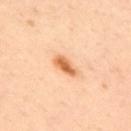follow-up = catalogued during a skin exam; not biopsied | patient = male, in their mid- to late 40s | tile lighting = cross-polarized illumination | lesion size = ≈3.5 mm | image = ~15 mm crop, total-body skin-cancer survey | location = the upper back.